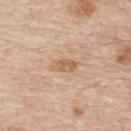Captured during whole-body skin photography for melanoma surveillance; the lesion was not biopsied. Approximately 3 mm at its widest. The tile uses white-light illumination. A 15 mm close-up tile from a total-body photography series done for melanoma screening. The lesion-visualizer software estimated a lesion area of about 4.5 mm² and an eccentricity of roughly 0.85. The software also gave a lesion color around L≈61 a*≈19 b*≈34 in CIELAB, roughly 9 lightness units darker than nearby skin, and a normalized lesion–skin contrast near 6. It also reported an automated nevus-likeness rating near 5 out of 100 and a lesion-detection confidence of about 100/100. From the upper back. A male patient, aged around 85 or older.